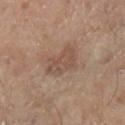Assessment: This lesion was catalogued during total-body skin photography and was not selected for biopsy. Acquisition and patient details: A region of skin cropped from a whole-body photographic capture, roughly 15 mm wide. The patient is a male in their 80s. Longest diameter approximately 4.5 mm. Automated tile analysis of the lesion measured a lesion color around L≈51 a*≈17 b*≈27 in CIELAB and about 8 CIELAB-L* units darker than the surrounding skin. And it measured a border-irregularity index near 2.5/10, internal color variation of about 2.5 on a 0–10 scale, and a peripheral color-asymmetry measure near 1. The tile uses white-light illumination. From the right lower leg.